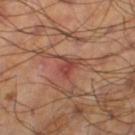Q: Was a biopsy performed?
A: no biopsy performed (imaged during a skin exam)
Q: Automated lesion metrics?
A: a mean CIELAB color near L≈44 a*≈25 b*≈27 and about 8 CIELAB-L* units darker than the surrounding skin
Q: Lesion location?
A: the leg
Q: How large is the lesion?
A: about 3.5 mm
Q: Illumination type?
A: cross-polarized
Q: What are the patient's age and sex?
A: male, in their 60s
Q: What kind of image is this?
A: total-body-photography crop, ~15 mm field of view